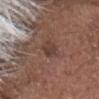Captured during whole-body skin photography for melanoma surveillance; the lesion was not biopsied. A region of skin cropped from a whole-body photographic capture, roughly 15 mm wide. Imaged with white-light lighting. The patient is a male aged 73–77. Located on the head or neck.